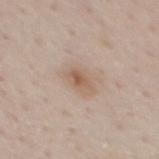Imaged during a routine full-body skin examination; the lesion was not biopsied and no histopathology is available.
The total-body-photography lesion software estimated a lesion area of about 4 mm², an outline eccentricity of about 0.8 (0 = round, 1 = elongated), and two-axis asymmetry of about 0.25. And it measured a mean CIELAB color near L≈58 a*≈17 b*≈29 and roughly 9 lightness units darker than nearby skin.
On the mid back.
A male patient, aged 48 to 52.
A 15 mm close-up extracted from a 3D total-body photography capture.